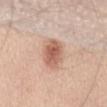subject: male, about 55 years old
size: ~4.5 mm (longest diameter)
location: the abdomen
tile lighting: white-light illumination
TBP lesion metrics: a lesion area of about 9.5 mm² and two-axis asymmetry of about 0.15; about 13 CIELAB-L* units darker than the surrounding skin and a normalized lesion–skin contrast near 8; a classifier nevus-likeness of about 95/100 and lesion-presence confidence of about 100/100
imaging modality: ~15 mm crop, total-body skin-cancer survey